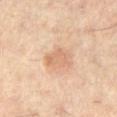| key | value |
|---|---|
| image | ~15 mm tile from a whole-body skin photo |
| location | the right thigh |
| patient | male, in their mid- to late 50s |
| lesion diameter | about 3.5 mm |
| TBP lesion metrics | a border-irregularity rating of about 3/10 and internal color variation of about 2.5 on a 0–10 scale; a classifier nevus-likeness of about 10/100 and lesion-presence confidence of about 100/100 |
| illumination | cross-polarized |